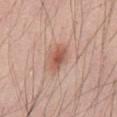This lesion was catalogued during total-body skin photography and was not selected for biopsy.